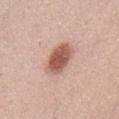Part of a total-body skin-imaging series; this lesion was reviewed on a skin check and was not flagged for biopsy. The lesion is on the mid back. Approximately 4 mm at its widest. Cropped from a total-body skin-imaging series; the visible field is about 15 mm. The tile uses white-light illumination. The subject is a male aged 33 to 37.